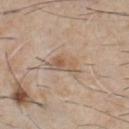Clinical impression:
Imaged during a routine full-body skin examination; the lesion was not biopsied and no histopathology is available.
Clinical summary:
On the chest. A male patient, aged 58–62. This image is a 15 mm lesion crop taken from a total-body photograph.